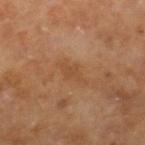Q: Is there a histopathology result?
A: imaged on a skin check; not biopsied
Q: What is the imaging modality?
A: 15 mm crop, total-body photography
Q: Automated lesion metrics?
A: a lesion area of about 5.5 mm², an eccentricity of roughly 0.9, and two-axis asymmetry of about 0.25
Q: Lesion location?
A: the right lower leg
Q: Illumination type?
A: cross-polarized illumination
Q: Patient demographics?
A: male, in their 60s
Q: What is the lesion's diameter?
A: ≈4 mm